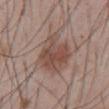| field | value |
|---|---|
| biopsy status | no biopsy performed (imaged during a skin exam) |
| image source | ~15 mm tile from a whole-body skin photo |
| site | the abdomen |
| subject | male, in their mid-50s |
| image-analysis metrics | a lesion area of about 17 mm² and two-axis asymmetry of about 0.25; a border-irregularity rating of about 3/10, a color-variation rating of about 4.5/10, and radial color variation of about 1.5; a lesion-detection confidence of about 100/100 |
| diameter | ~5.5 mm (longest diameter) |
| lighting | white-light illumination |